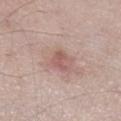Clinical impression:
Part of a total-body skin-imaging series; this lesion was reviewed on a skin check and was not flagged for biopsy.
Acquisition and patient details:
The lesion is located on the right lower leg. A male subject, in their 60s. Imaged with white-light lighting. Automated tile analysis of the lesion measured a footprint of about 3.5 mm², a shape eccentricity near 0.75, and two-axis asymmetry of about 0.35. Measured at roughly 2.5 mm in maximum diameter. A 15 mm crop from a total-body photograph taken for skin-cancer surveillance.